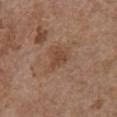  biopsy_status: not biopsied; imaged during a skin examination
  lesion_size:
    long_diameter_mm_approx: 2.5
  site: chest
  image:
    source: total-body photography crop
    field_of_view_mm: 15
  patient:
    sex: female
    age_approx: 65